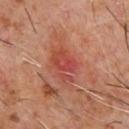Q: Was this lesion biopsied?
A: imaged on a skin check; not biopsied
Q: What lighting was used for the tile?
A: cross-polarized illumination
Q: How large is the lesion?
A: about 4.5 mm
Q: What is the imaging modality?
A: ~15 mm tile from a whole-body skin photo
Q: Where on the body is the lesion?
A: the chest
Q: Who is the patient?
A: male, roughly 60 years of age